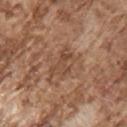The subject is a male in their mid-70s. Cropped from a total-body skin-imaging series; the visible field is about 15 mm. The lesion is located on the right upper arm. The total-body-photography lesion software estimated a footprint of about 7 mm², an eccentricity of roughly 0.85, and two-axis asymmetry of about 0.45. It also reported a border-irregularity rating of about 5/10, a color-variation rating of about 4/10, and radial color variation of about 1.5. It also reported an automated nevus-likeness rating near 0 out of 100 and lesion-presence confidence of about 95/100. Longest diameter approximately 4.5 mm. This is a white-light tile.